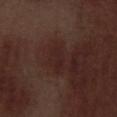| key | value |
|---|---|
| follow-up | catalogued during a skin exam; not biopsied |
| lesion diameter | ≈3 mm |
| site | the left thigh |
| acquisition | total-body-photography crop, ~15 mm field of view |
| patient | male, about 70 years old |